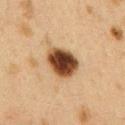Clinical impression: The lesion was tiled from a total-body skin photograph and was not biopsied. Image and clinical context: A female subject approximately 40 years of age. A 15 mm close-up tile from a total-body photography series done for melanoma screening. Located on the right upper arm. The lesion's longest dimension is about 4 mm. Captured under cross-polarized illumination.